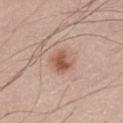Q: Is there a histopathology result?
A: no biopsy performed (imaged during a skin exam)
Q: How was the tile lit?
A: white-light illumination
Q: What are the patient's age and sex?
A: male, in their 40s
Q: How was this image acquired?
A: total-body-photography crop, ~15 mm field of view
Q: How large is the lesion?
A: ≈3 mm
Q: What is the anatomic site?
A: the leg